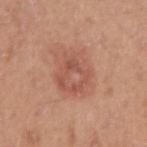Q: Was a biopsy performed?
A: total-body-photography surveillance lesion; no biopsy
Q: How large is the lesion?
A: ~4.5 mm (longest diameter)
Q: What are the patient's age and sex?
A: male, in their 40s
Q: Illumination type?
A: white-light illumination
Q: Lesion location?
A: the right upper arm
Q: What kind of image is this?
A: total-body-photography crop, ~15 mm field of view